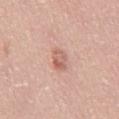Case summary:
- follow-up: catalogued during a skin exam; not biopsied
- image source: ~15 mm crop, total-body skin-cancer survey
- automated lesion analysis: a mean CIELAB color near L≈62 a*≈24 b*≈27 and a lesion–skin lightness drop of about 10; border irregularity of about 3.5 on a 0–10 scale, internal color variation of about 4.5 on a 0–10 scale, and a peripheral color-asymmetry measure near 1.5; a classifier nevus-likeness of about 10/100 and a lesion-detection confidence of about 100/100
- size: ~3.5 mm (longest diameter)
- location: the abdomen
- subject: male, aged around 50
- tile lighting: white-light illumination